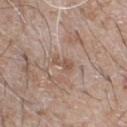– biopsy status: imaged on a skin check; not biopsied
– image source: 15 mm crop, total-body photography
– patient: male, in their mid- to late 60s
– location: the chest
– image-analysis metrics: a lesion color around L≈53 a*≈18 b*≈27 in CIELAB, about 7 CIELAB-L* units darker than the surrounding skin, and a lesion-to-skin contrast of about 6 (normalized; higher = more distinct); a border-irregularity index near 4/10, a within-lesion color-variation index near 0.5/10, and a peripheral color-asymmetry measure near 0
– lighting: white-light illumination